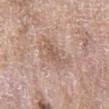The lesion is on the left thigh.
A region of skin cropped from a whole-body photographic capture, roughly 15 mm wide.
This is a white-light tile.
The total-body-photography lesion software estimated a footprint of about 5.5 mm², a shape eccentricity near 0.95, and two-axis asymmetry of about 0.4. And it measured a lesion color around L≈57 a*≈18 b*≈26 in CIELAB and a lesion-to-skin contrast of about 6 (normalized; higher = more distinct).
The subject is a female aged around 60.
Longest diameter approximately 4.5 mm.